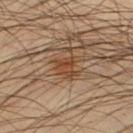notes=total-body-photography surveillance lesion; no biopsy
image=~15 mm tile from a whole-body skin photo
lighting=cross-polarized illumination
subject=male, roughly 45 years of age
site=the right thigh